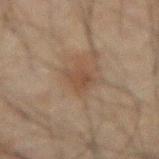{
  "biopsy_status": "not biopsied; imaged during a skin examination",
  "image": {
    "source": "total-body photography crop",
    "field_of_view_mm": 15
  },
  "lighting": "cross-polarized",
  "automated_metrics": {
    "cielab_L": 35,
    "cielab_a": 13,
    "cielab_b": 23,
    "vs_skin_darker_L": 5.0,
    "border_irregularity_0_10": 3.5,
    "color_variation_0_10": 2.0,
    "peripheral_color_asymmetry": 0.5
  },
  "patient": {
    "sex": "male",
    "age_approx": 60
  },
  "lesion_size": {
    "long_diameter_mm_approx": 2.5
  },
  "site": "abdomen"
}